A male subject, roughly 25 years of age · a region of skin cropped from a whole-body photographic capture, roughly 15 mm wide · located on the chest.
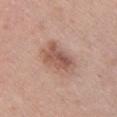On excision, pathology confirmed a benign skin lesion: dysplastic (Clark) nevus.